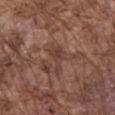This lesion was catalogued during total-body skin photography and was not selected for biopsy.
The lesion-visualizer software estimated a nevus-likeness score of about 0/100 and lesion-presence confidence of about 90/100.
The lesion is located on the chest.
About 4 mm across.
A lesion tile, about 15 mm wide, cut from a 3D total-body photograph.
Captured under white-light illumination.
A male subject, aged 73 to 77.